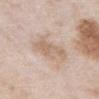workup — imaged on a skin check; not biopsied | automated lesion analysis — a lesion area of about 11 mm², a shape eccentricity near 0.7, and a symmetry-axis asymmetry near 0.3; a lesion color around L≈65 a*≈15 b*≈28 in CIELAB and a normalized border contrast of about 6; a detector confidence of about 100 out of 100 that the crop contains a lesion | patient — male, about 55 years old | image source — ~15 mm crop, total-body skin-cancer survey | lesion size — ≈4.5 mm | anatomic site — the chest.